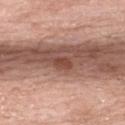workup: no biopsy performed (imaged during a skin exam)
subject: female, aged 68–72
size: ≈2.5 mm
acquisition: 15 mm crop, total-body photography
location: the upper back